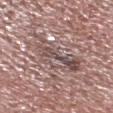Recorded during total-body skin imaging; not selected for excision or biopsy.
A 15 mm close-up tile from a total-body photography series done for melanoma screening.
The subject is a male aged approximately 65.
The lesion is located on the head or neck.